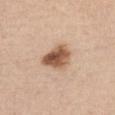Clinical impression:
The lesion was photographed on a routine skin check and not biopsied; there is no pathology result.
Image and clinical context:
This image is a 15 mm lesion crop taken from a total-body photograph. The lesion's longest dimension is about 4.5 mm. The lesion is located on the left upper arm. The tile uses white-light illumination. Automated image analysis of the tile measured a color-variation rating of about 7/10. A male subject aged approximately 75.